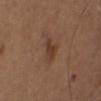Notes:
• image source · ~15 mm crop, total-body skin-cancer survey
• patient · female, aged 38–42
• location · the upper back